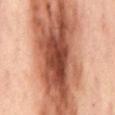Cropped from a total-body skin-imaging series; the visible field is about 15 mm. The total-body-photography lesion software estimated a footprint of about 135 mm², an eccentricity of roughly 0.95, and a symmetry-axis asymmetry near 0.25. It also reported a lesion–skin lightness drop of about 15 and a lesion-to-skin contrast of about 10.5 (normalized; higher = more distinct). And it measured a border-irregularity rating of about 4/10, a within-lesion color-variation index near 8/10, and a peripheral color-asymmetry measure near 2.5. The analysis additionally found a classifier nevus-likeness of about 90/100. On the mid back. A female patient, aged 53–57. Approximately 23.5 mm at its widest.